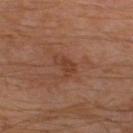workup: no biopsy performed (imaged during a skin exam); image: ~15 mm crop, total-body skin-cancer survey; patient: male, aged 58 to 62; body site: the leg.